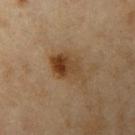Q: Was a biopsy performed?
A: total-body-photography surveillance lesion; no biopsy
Q: What are the patient's age and sex?
A: female, aged around 60
Q: Lesion size?
A: ~4.5 mm (longest diameter)
Q: Automated lesion metrics?
A: an outline eccentricity of about 0.75 (0 = round, 1 = elongated) and two-axis asymmetry of about 0.3; an average lesion color of about L≈38 a*≈16 b*≈30 (CIELAB) and roughly 9 lightness units darker than nearby skin; an automated nevus-likeness rating near 100 out of 100 and a lesion-detection confidence of about 100/100
Q: What is the anatomic site?
A: the left upper arm
Q: What is the imaging modality?
A: total-body-photography crop, ~15 mm field of view
Q: What lighting was used for the tile?
A: cross-polarized